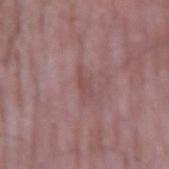follow-up = imaged on a skin check; not biopsied | diameter = ~3 mm (longest diameter) | acquisition = total-body-photography crop, ~15 mm field of view | patient = male, roughly 65 years of age | site = the right forearm | illumination = white-light illumination.